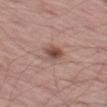This lesion was catalogued during total-body skin photography and was not selected for biopsy.
Cropped from a total-body skin-imaging series; the visible field is about 15 mm.
The subject is a male roughly 60 years of age.
This is a white-light tile.
From the left thigh.
The recorded lesion diameter is about 2.5 mm.